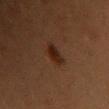- notes — no biopsy performed (imaged during a skin exam)
- patient — female, aged 78–82
- automated metrics — a lesion–skin lightness drop of about 7 and a normalized lesion–skin contrast near 9; a border-irregularity index near 3/10 and a within-lesion color-variation index near 2.5/10
- lesion size — ~3.5 mm (longest diameter)
- tile lighting — cross-polarized
- imaging modality — 15 mm crop, total-body photography
- location — the upper back